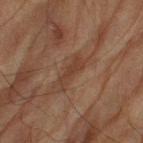Clinical impression: This lesion was catalogued during total-body skin photography and was not selected for biopsy. Image and clinical context: The lesion is located on the right thigh. A male subject aged approximately 85 or older. Measured at roughly 4.5 mm in maximum diameter. Cropped from a whole-body photographic skin survey; the tile spans about 15 mm. The tile uses cross-polarized illumination. The total-body-photography lesion software estimated a footprint of about 4.5 mm². And it measured an average lesion color of about L≈33 a*≈18 b*≈25 (CIELAB) and a normalized lesion–skin contrast near 6.